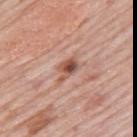Findings:
- biopsy status: total-body-photography surveillance lesion; no biopsy
- body site: the mid back
- patient: male, aged approximately 80
- TBP lesion metrics: a lesion area of about 4.5 mm² and two-axis asymmetry of about 0.3; a mean CIELAB color near L≈52 a*≈23 b*≈28, about 13 CIELAB-L* units darker than the surrounding skin, and a normalized border contrast of about 9; radial color variation of about 1.5
- lesion diameter: ~3 mm (longest diameter)
- lighting: white-light illumination
- acquisition: 15 mm crop, total-body photography